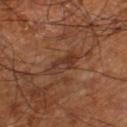workup=catalogued during a skin exam; not biopsied
lesion size=≈4.5 mm
image source=15 mm crop, total-body photography
tile lighting=cross-polarized illumination
patient=aged approximately 65
location=the leg